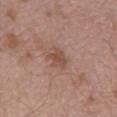The lesion was tiled from a total-body skin photograph and was not biopsied. A lesion tile, about 15 mm wide, cut from a 3D total-body photograph. Imaged with white-light lighting. From the lower back. Automated image analysis of the tile measured a mean CIELAB color near L≈50 a*≈20 b*≈27, roughly 8 lightness units darker than nearby skin, and a normalized lesion–skin contrast near 6.5. It also reported a border-irregularity rating of about 4/10, a color-variation rating of about 2/10, and a peripheral color-asymmetry measure near 0.5. A male subject in their mid- to late 50s. The recorded lesion diameter is about 3.5 mm.